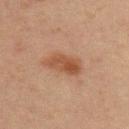workup=total-body-photography surveillance lesion; no biopsy
size=about 5 mm
patient=male, in their 30s
acquisition=~15 mm tile from a whole-body skin photo
site=the upper back